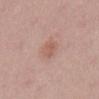notes=catalogued during a skin exam; not biopsied | body site=the abdomen | diameter=~3 mm (longest diameter) | tile lighting=white-light illumination | subject=male, aged 53 to 57 | acquisition=~15 mm tile from a whole-body skin photo.